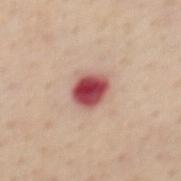Impression:
No biopsy was performed on this lesion — it was imaged during a full skin examination and was not determined to be concerning.
Background:
A 15 mm close-up tile from a total-body photography series done for melanoma screening. About 3.5 mm across. This is a white-light tile. A female patient, aged 48–52. The total-body-photography lesion software estimated a footprint of about 7.5 mm², a shape eccentricity near 0.55, and two-axis asymmetry of about 0.15. It also reported a mean CIELAB color near L≈49 a*≈33 b*≈24, roughly 21 lightness units darker than nearby skin, and a normalized border contrast of about 13.5. The analysis additionally found a classifier nevus-likeness of about 0/100 and lesion-presence confidence of about 100/100. From the mid back.